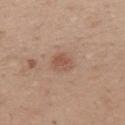follow-up: no biopsy performed (imaged during a skin exam)
size: about 2.5 mm
illumination: white-light
location: the mid back
image source: 15 mm crop, total-body photography
patient: male, about 35 years old